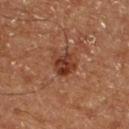No biopsy was performed on this lesion — it was imaged during a full skin examination and was not determined to be concerning. Captured under cross-polarized illumination. A 15 mm close-up tile from a total-body photography series done for melanoma screening. A male patient about 65 years old. The lesion is located on the left lower leg. Approximately 3 mm at its widest.